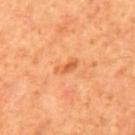- biopsy status · catalogued during a skin exam; not biopsied
- TBP lesion metrics · an average lesion color of about L≈60 a*≈30 b*≈45 (CIELAB), a lesion–skin lightness drop of about 9, and a normalized border contrast of about 6.5; a border-irregularity rating of about 4.5/10, internal color variation of about 0 on a 0–10 scale, and peripheral color asymmetry of about 0; an automated nevus-likeness rating near 0 out of 100 and a detector confidence of about 100 out of 100 that the crop contains a lesion
- patient · male, about 65 years old
- diameter · ≈3 mm
- location · the mid back
- lighting · cross-polarized illumination
- acquisition · total-body-photography crop, ~15 mm field of view